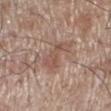On the leg. A male patient aged 68–72. Cropped from a total-body skin-imaging series; the visible field is about 15 mm.